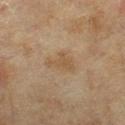| feature | finding |
|---|---|
| biopsy status | total-body-photography surveillance lesion; no biopsy |
| size | about 3.5 mm |
| acquisition | ~15 mm tile from a whole-body skin photo |
| location | the right lower leg |
| subject | female, aged around 60 |
| image-analysis metrics | a classifier nevus-likeness of about 0/100 |